This image is a 15 mm lesion crop taken from a total-body photograph, located on the left lower leg, a female subject aged 63–67: 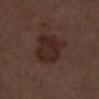The biopsy diagnosis was a squamous cell carcinoma in situ (malignant).